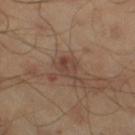Clinical impression: No biopsy was performed on this lesion — it was imaged during a full skin examination and was not determined to be concerning. Clinical summary: The lesion is located on the right thigh. The lesion-visualizer software estimated roughly 7 lightness units darker than nearby skin and a normalized border contrast of about 6.5. And it measured a border-irregularity index near 2.5/10, a within-lesion color-variation index near 3.5/10, and radial color variation of about 1.5. This image is a 15 mm lesion crop taken from a total-body photograph. The tile uses cross-polarized illumination. A male patient aged 43 to 47. About 3.5 mm across.